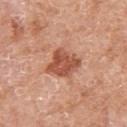A close-up tile cropped from a whole-body skin photograph, about 15 mm across.
About 4 mm across.
The tile uses white-light illumination.
A female subject, aged 73 to 77.
On the right upper arm.
The total-body-photography lesion software estimated an area of roughly 11 mm², an eccentricity of roughly 0.6, and a symmetry-axis asymmetry near 0.2. And it measured a detector confidence of about 100 out of 100 that the crop contains a lesion.